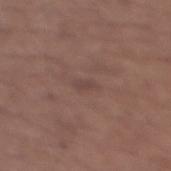The lesion was photographed on a routine skin check and not biopsied; there is no pathology result. Captured under white-light illumination. The lesion-visualizer software estimated a mean CIELAB color near L≈43 a*≈17 b*≈20, a lesion–skin lightness drop of about 5, and a lesion-to-skin contrast of about 5 (normalized; higher = more distinct). It also reported a border-irregularity index near 3.5/10, a within-lesion color-variation index near 0/10, and radial color variation of about 0. And it measured a classifier nevus-likeness of about 0/100. The recorded lesion diameter is about 2.5 mm. A female patient, aged around 65. From the upper back. A 15 mm close-up tile from a total-body photography series done for melanoma screening.